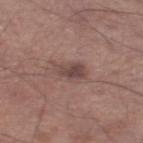Impression:
The lesion was tiled from a total-body skin photograph and was not biopsied.
Context:
Longest diameter approximately 3 mm. Automated tile analysis of the lesion measured roughly 10 lightness units darker than nearby skin and a normalized border contrast of about 7.5. The software also gave a border-irregularity rating of about 3/10 and a peripheral color-asymmetry measure near 1. The analysis additionally found a nevus-likeness score of about 15/100 and lesion-presence confidence of about 100/100. A region of skin cropped from a whole-body photographic capture, roughly 15 mm wide. Located on the left lower leg. The subject is a male aged 63–67. This is a white-light tile.